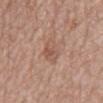The lesion was photographed on a routine skin check and not biopsied; there is no pathology result. The lesion is on the mid back. Longest diameter approximately 4.5 mm. The lesion-visualizer software estimated a footprint of about 6 mm², an eccentricity of roughly 0.9, and two-axis asymmetry of about 0.3. And it measured a border-irregularity index near 3.5/10, internal color variation of about 3 on a 0–10 scale, and radial color variation of about 1. A lesion tile, about 15 mm wide, cut from a 3D total-body photograph. A male subject, aged approximately 80.